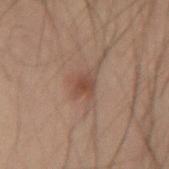Captured during whole-body skin photography for melanoma surveillance; the lesion was not biopsied. From the right forearm. A 15 mm close-up tile from a total-body photography series done for melanoma screening. This is a cross-polarized tile. Automated image analysis of the tile measured a lesion area of about 5.5 mm² and a shape eccentricity near 0.5. The software also gave a mean CIELAB color near L≈41 a*≈17 b*≈23 and roughly 8 lightness units darker than nearby skin. It also reported a lesion-detection confidence of about 100/100. A male patient, about 40 years old. About 3 mm across.